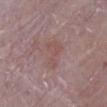The lesion was photographed on a routine skin check and not biopsied; there is no pathology result.
A female subject, aged 63 to 67.
On the leg.
The lesion-visualizer software estimated a lesion color around L≈51 a*≈20 b*≈20 in CIELAB and a lesion-to-skin contrast of about 4.5 (normalized; higher = more distinct). It also reported a classifier nevus-likeness of about 0/100 and a detector confidence of about 100 out of 100 that the crop contains a lesion.
A lesion tile, about 15 mm wide, cut from a 3D total-body photograph.
The tile uses white-light illumination.